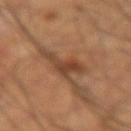Recorded during total-body skin imaging; not selected for excision or biopsy.